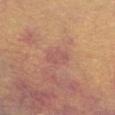notes — total-body-photography surveillance lesion; no biopsy
patient — female, aged approximately 50
automated lesion analysis — a lesion color around L≈55 a*≈25 b*≈25 in CIELAB and a normalized border contrast of about 5.5; a border-irregularity rating of about 3/10, a color-variation rating of about 1.5/10, and a peripheral color-asymmetry measure near 0.5
site — the chest
lighting — white-light
image source — total-body-photography crop, ~15 mm field of view